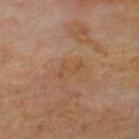diameter=about 3.5 mm; location=the upper back; subject=male, aged approximately 70; lighting=cross-polarized; acquisition=~15 mm tile from a whole-body skin photo; image-analysis metrics=an average lesion color of about L≈39 a*≈17 b*≈28 (CIELAB), roughly 4 lightness units darker than nearby skin, and a normalized lesion–skin contrast near 5.